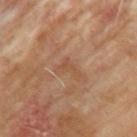Case summary:
• illumination · cross-polarized
• body site · the left upper arm
• acquisition · total-body-photography crop, ~15 mm field of view
• image-analysis metrics · an average lesion color of about L≈48 a*≈20 b*≈31 (CIELAB); a detector confidence of about 100 out of 100 that the crop contains a lesion
• patient · male, aged 63–67
• lesion size · ≈3 mm